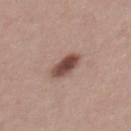follow-up = imaged on a skin check; not biopsied
anatomic site = the upper back
patient = female, aged 23 to 27
diameter = ≈3.5 mm
tile lighting = white-light illumination
image = ~15 mm crop, total-body skin-cancer survey